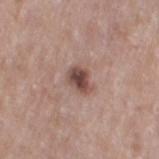workup = no biopsy performed (imaged during a skin exam) | location = the leg | illumination = white-light illumination | image source = ~15 mm tile from a whole-body skin photo | lesion size = ≈3 mm | subject = female, about 60 years old | automated metrics = an area of roughly 5 mm², an eccentricity of roughly 0.65, and a symmetry-axis asymmetry near 0.25; internal color variation of about 4 on a 0–10 scale and a peripheral color-asymmetry measure near 1; an automated nevus-likeness rating near 75 out of 100.